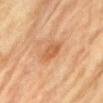follow-up: catalogued during a skin exam; not biopsied
patient: female, aged 78–82
image: total-body-photography crop, ~15 mm field of view
location: the abdomen
illumination: cross-polarized illumination
diameter: ≈3 mm